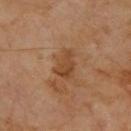Part of a total-body skin-imaging series; this lesion was reviewed on a skin check and was not flagged for biopsy. Cropped from a whole-body photographic skin survey; the tile spans about 15 mm. Located on the upper back. A male subject roughly 70 years of age.